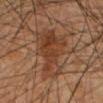Notes:
- size · ≈6 mm
- body site · the mid back
- subject · male, approximately 60 years of age
- illumination · cross-polarized illumination
- image-analysis metrics · a mean CIELAB color near L≈35 a*≈19 b*≈28 and a lesion–skin lightness drop of about 7; a border-irregularity index near 5.5/10; lesion-presence confidence of about 100/100
- image · ~15 mm crop, total-body skin-cancer survey